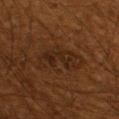A male subject aged 58–62. Measured at roughly 5 mm in maximum diameter. Imaged with cross-polarized lighting. The total-body-photography lesion software estimated a mean CIELAB color near L≈20 a*≈15 b*≈23 and roughly 4 lightness units darker than nearby skin. And it measured a within-lesion color-variation index near 3/10 and radial color variation of about 1. On the left lower leg. A close-up tile cropped from a whole-body skin photograph, about 15 mm across.